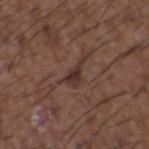biopsy_status: not biopsied; imaged during a skin examination
lighting: white-light
automated_metrics:
  eccentricity: 0.8
  shape_asymmetry: 0.6
  nevus_likeness_0_100: 35
  lesion_detection_confidence_0_100: 80
site: upper back
lesion_size:
  long_diameter_mm_approx: 3.5
image:
  source: total-body photography crop
  field_of_view_mm: 15
patient:
  sex: male
  age_approx: 50